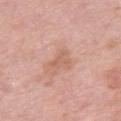Clinical impression:
The lesion was tiled from a total-body skin photograph and was not biopsied.
Context:
A 15 mm close-up extracted from a 3D total-body photography capture. The subject is a female aged 68–72. Approximately 2.5 mm at its widest. Automated tile analysis of the lesion measured an area of roughly 3.5 mm², a shape eccentricity near 0.7, and a symmetry-axis asymmetry near 0.5. And it measured a mean CIELAB color near L≈61 a*≈23 b*≈30 and roughly 7 lightness units darker than nearby skin. And it measured border irregularity of about 5.5 on a 0–10 scale, a within-lesion color-variation index near 0.5/10, and radial color variation of about 0. It also reported lesion-presence confidence of about 100/100. On the left thigh.